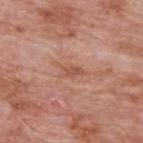Findings:
– follow-up — catalogued during a skin exam; not biopsied
– subject — male, aged 58–62
– lesion diameter — about 3.5 mm
– illumination — white-light illumination
– anatomic site — the upper back
– imaging modality — 15 mm crop, total-body photography